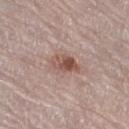notes: no biopsy performed (imaged during a skin exam) | lighting: white-light | acquisition: total-body-photography crop, ~15 mm field of view | body site: the right thigh | patient: female, approximately 65 years of age | lesion diameter: ≈3.5 mm | TBP lesion metrics: a lesion area of about 6.5 mm², an eccentricity of roughly 0.8, and a shape-asymmetry score of about 0.3 (0 = symmetric); an average lesion color of about L≈52 a*≈20 b*≈25 (CIELAB), a lesion–skin lightness drop of about 11, and a lesion-to-skin contrast of about 8 (normalized; higher = more distinct).